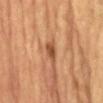The lesion was photographed on a routine skin check and not biopsied; there is no pathology result. Automated image analysis of the tile measured an average lesion color of about L≈44 a*≈21 b*≈32 (CIELAB) and a lesion-to-skin contrast of about 8 (normalized; higher = more distinct). The analysis additionally found a nevus-likeness score of about 5/100 and a lesion-detection confidence of about 100/100. The lesion's longest dimension is about 3 mm. This image is a 15 mm lesion crop taken from a total-body photograph. A male patient aged approximately 85 or older. Located on the abdomen.